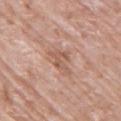Q: Was a biopsy performed?
A: total-body-photography surveillance lesion; no biopsy
Q: Lesion location?
A: the upper back
Q: Who is the patient?
A: female, aged 83 or older
Q: How was this image acquired?
A: ~15 mm tile from a whole-body skin photo
Q: Lesion size?
A: ≈4 mm